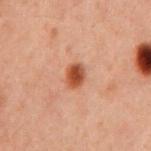Impression:
Imaged during a routine full-body skin examination; the lesion was not biopsied and no histopathology is available.
Acquisition and patient details:
Cropped from a whole-body photographic skin survey; the tile spans about 15 mm. On the chest. Approximately 2.5 mm at its widest. The tile uses cross-polarized illumination. A male patient in their 60s.